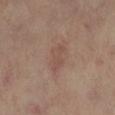workup = imaged on a skin check; not biopsied | location = the left lower leg | patient = female, approximately 65 years of age | imaging modality = 15 mm crop, total-body photography.